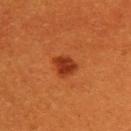No biopsy was performed on this lesion — it was imaged during a full skin examination and was not determined to be concerning.
A 15 mm close-up extracted from a 3D total-body photography capture.
The patient is a female roughly 30 years of age.
The lesion is on the upper back.
The total-body-photography lesion software estimated a lesion color around L≈36 a*≈33 b*≈39 in CIELAB and a lesion–skin lightness drop of about 11.